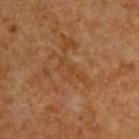Notes:
- follow-up: total-body-photography surveillance lesion; no biopsy
- subject: male, approximately 60 years of age
- anatomic site: the back
- image source: ~15 mm tile from a whole-body skin photo